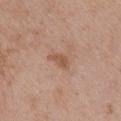biopsy status — no biopsy performed (imaged during a skin exam) | diameter — ≈3 mm | location — the chest | illumination — white-light illumination | automated lesion analysis — a lesion area of about 3.5 mm², an eccentricity of roughly 0.85, and two-axis asymmetry of about 0.4; a border-irregularity index near 3.5/10, internal color variation of about 1.5 on a 0–10 scale, and a peripheral color-asymmetry measure near 0.5 | acquisition — ~15 mm tile from a whole-body skin photo | subject — female, aged approximately 65.